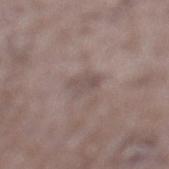automated lesion analysis: a lesion color around L≈50 a*≈13 b*≈18 in CIELAB, about 7 CIELAB-L* units darker than the surrounding skin, and a lesion-to-skin contrast of about 5.5 (normalized; higher = more distinct); a border-irregularity index near 2/10, internal color variation of about 2 on a 0–10 scale, and radial color variation of about 0.5; an automated nevus-likeness rating near 0 out of 100 and a lesion-detection confidence of about 85/100
image source: ~15 mm crop, total-body skin-cancer survey
tile lighting: white-light illumination
patient: male, aged 68–72
anatomic site: the left lower leg
size: about 3 mm